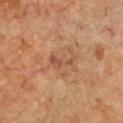follow-up: catalogued during a skin exam; not biopsied